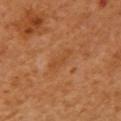Captured during whole-body skin photography for melanoma surveillance; the lesion was not biopsied. A close-up tile cropped from a whole-body skin photograph, about 15 mm across. Located on the right upper arm. A female patient, about 55 years old. Captured under cross-polarized illumination. The recorded lesion diameter is about 3.5 mm.